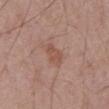<case>
  <biopsy_status>not biopsied; imaged during a skin examination</biopsy_status>
  <image>
    <source>total-body photography crop</source>
    <field_of_view_mm>15</field_of_view_mm>
  </image>
  <patient>
    <sex>male</sex>
    <age_approx>70</age_approx>
  </patient>
  <lesion_size>
    <long_diameter_mm_approx>3.0</long_diameter_mm_approx>
  </lesion_size>
  <automated_metrics>
    <area_mm2_approx>5.0</area_mm2_approx>
    <cielab_L>52</cielab_L>
    <cielab_a>21</cielab_a>
    <cielab_b>27</cielab_b>
    <vs_skin_darker_L>7.0</vs_skin_darker_L>
    <vs_skin_contrast_norm>6.0</vs_skin_contrast_norm>
    <border_irregularity_0_10>2.5</border_irregularity_0_10>
    <peripheral_color_asymmetry>0.5</peripheral_color_asymmetry>
    <nevus_likeness_0_100>10</nevus_likeness_0_100>
    <lesion_detection_confidence_0_100>100</lesion_detection_confidence_0_100>
  </automated_metrics>
  <site>abdomen</site>
</case>